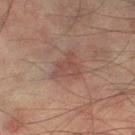Assessment:
Recorded during total-body skin imaging; not selected for excision or biopsy.
Image and clinical context:
A roughly 15 mm field-of-view crop from a total-body skin photograph. Automated image analysis of the tile measured an area of roughly 7 mm², an eccentricity of roughly 0.5, and two-axis asymmetry of about 0.45. It also reported border irregularity of about 4.5 on a 0–10 scale, a color-variation rating of about 2/10, and a peripheral color-asymmetry measure near 0.5. It also reported a classifier nevus-likeness of about 0/100 and a detector confidence of about 100 out of 100 that the crop contains a lesion. About 3.5 mm across. A male patient aged approximately 75. From the left thigh.